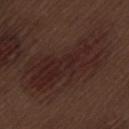Impression: Part of a total-body skin-imaging series; this lesion was reviewed on a skin check and was not flagged for biopsy. Image and clinical context: The lesion's longest dimension is about 10.5 mm. Imaged with white-light lighting. Automated image analysis of the tile measured an area of roughly 32 mm² and an outline eccentricity of about 0.9 (0 = round, 1 = elongated). It also reported lesion-presence confidence of about 90/100. On the leg. This image is a 15 mm lesion crop taken from a total-body photograph. A male subject, approximately 70 years of age.